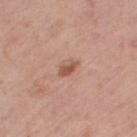– follow-up · total-body-photography surveillance lesion; no biopsy
– lighting · white-light
– lesion diameter · ≈2.5 mm
– image · ~15 mm tile from a whole-body skin photo
– patient · male, about 75 years old
– location · the leg